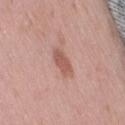notes = imaged on a skin check; not biopsied.